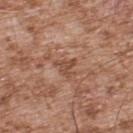Assessment:
The lesion was photographed on a routine skin check and not biopsied; there is no pathology result.
Clinical summary:
Cropped from a whole-body photographic skin survey; the tile spans about 15 mm. Located on the upper back. Measured at roughly 2.5 mm in maximum diameter. This is a white-light tile. The patient is a male in their mid-50s.